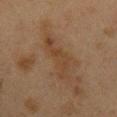Acquisition and patient details:
The lesion is on the front of the torso. A 15 mm close-up tile from a total-body photography series done for melanoma screening. A female patient, about 40 years old.